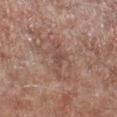patient=male, in their mid- to late 50s | automated lesion analysis=a border-irregularity index near 5.5/10, a within-lesion color-variation index near 1.5/10, and a peripheral color-asymmetry measure near 0.5; an automated nevus-likeness rating near 0 out of 100 | image=~15 mm crop, total-body skin-cancer survey | location=the right lower leg | lighting=white-light | diameter=~4 mm (longest diameter).